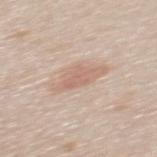Part of a total-body skin-imaging series; this lesion was reviewed on a skin check and was not flagged for biopsy. A male patient roughly 40 years of age. This is a white-light tile. Located on the mid back. A roughly 15 mm field-of-view crop from a total-body skin photograph. Longest diameter approximately 3 mm. The lesion-visualizer software estimated a footprint of about 3.5 mm² and a shape-asymmetry score of about 0.35 (0 = symmetric).